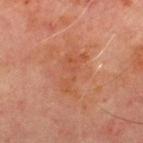Q: Is there a histopathology result?
A: catalogued during a skin exam; not biopsied
Q: How was this image acquired?
A: 15 mm crop, total-body photography
Q: Lesion size?
A: ≈5 mm
Q: How was the tile lit?
A: cross-polarized
Q: Lesion location?
A: the chest
Q: What are the patient's age and sex?
A: male, aged 68–72